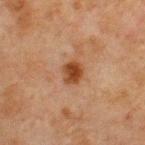notes — total-body-photography surveillance lesion; no biopsy
subject — male, roughly 65 years of age
imaging modality — total-body-photography crop, ~15 mm field of view
lesion size — ≈2.5 mm
anatomic site — the chest
lighting — cross-polarized
TBP lesion metrics — a lesion–skin lightness drop of about 11 and a normalized border contrast of about 10; a nevus-likeness score of about 95/100